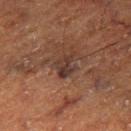| feature | finding |
|---|---|
| workup | imaged on a skin check; not biopsied |
| image source | ~15 mm tile from a whole-body skin photo |
| lighting | cross-polarized illumination |
| size | ~3.5 mm (longest diameter) |
| location | the right thigh |
| patient | male, approximately 75 years of age |
| automated metrics | a lesion area of about 5 mm² and an outline eccentricity of about 0.75 (0 = round, 1 = elongated); a lesion color around L≈27 a*≈16 b*≈19 in CIELAB, about 7 CIELAB-L* units darker than the surrounding skin, and a normalized border contrast of about 9 |